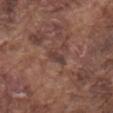No biopsy was performed on this lesion — it was imaged during a full skin examination and was not determined to be concerning. Located on the mid back. Longest diameter approximately 3 mm. Imaged with white-light lighting. Automated tile analysis of the lesion measured a footprint of about 3.5 mm² and a shape-asymmetry score of about 0.35 (0 = symmetric). It also reported a lesion color around L≈39 a*≈18 b*≈22 in CIELAB. And it measured a border-irregularity rating of about 3.5/10, a within-lesion color-variation index near 3/10, and a peripheral color-asymmetry measure near 1.5. A region of skin cropped from a whole-body photographic capture, roughly 15 mm wide. The subject is a male aged around 75.